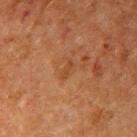{
  "biopsy_status": "not biopsied; imaged during a skin examination",
  "site": "right upper arm",
  "image": {
    "source": "total-body photography crop",
    "field_of_view_mm": 15
  },
  "patient": {
    "sex": "male",
    "age_approx": 60
  },
  "lighting": "cross-polarized"
}